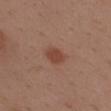Impression: The lesion was tiled from a total-body skin photograph and was not biopsied. Acquisition and patient details: A region of skin cropped from a whole-body photographic capture, roughly 15 mm wide. The lesion is on the upper back. Captured under white-light illumination. The subject is a male aged around 40.